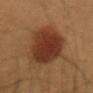This lesion was catalogued during total-body skin photography and was not selected for biopsy. Imaged with cross-polarized lighting. A roughly 15 mm field-of-view crop from a total-body skin photograph. An algorithmic analysis of the crop reported a lesion area of about 25 mm², an outline eccentricity of about 0.5 (0 = round, 1 = elongated), and a symmetry-axis asymmetry near 0.15. The analysis additionally found border irregularity of about 1.5 on a 0–10 scale, a color-variation rating of about 4/10, and radial color variation of about 1. And it measured lesion-presence confidence of about 100/100. The lesion is located on the right upper arm. A male subject, aged around 40. Measured at roughly 6 mm in maximum diameter.